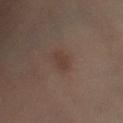Impression: Recorded during total-body skin imaging; not selected for excision or biopsy. Background: A region of skin cropped from a whole-body photographic capture, roughly 15 mm wide. Imaged with cross-polarized lighting. Located on the left lower leg. The recorded lesion diameter is about 2.5 mm. A female patient about 55 years old.